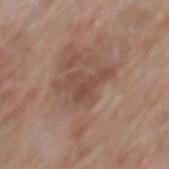The lesion was photographed on a routine skin check and not biopsied; there is no pathology result. A lesion tile, about 15 mm wide, cut from a 3D total-body photograph. This is a white-light tile. On the mid back. Automated tile analysis of the lesion measured a footprint of about 9 mm², a shape eccentricity near 0.8, and two-axis asymmetry of about 0.55. The software also gave a lesion color around L≈47 a*≈18 b*≈26 in CIELAB and a lesion-to-skin contrast of about 6 (normalized; higher = more distinct). And it measured a border-irregularity index near 6.5/10, internal color variation of about 3.5 on a 0–10 scale, and peripheral color asymmetry of about 1.5. A male patient, aged around 60. The lesion's longest dimension is about 5.5 mm.